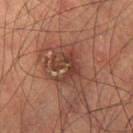Part of a total-body skin-imaging series; this lesion was reviewed on a skin check and was not flagged for biopsy.
A region of skin cropped from a whole-body photographic capture, roughly 15 mm wide.
Approximately 3.5 mm at its widest.
The patient is a male aged 68 to 72.
From the right thigh.